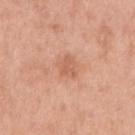Captured during whole-body skin photography for melanoma surveillance; the lesion was not biopsied.
From the right upper arm.
Automated tile analysis of the lesion measured an outline eccentricity of about 0.4 (0 = round, 1 = elongated) and a shape-asymmetry score of about 0.25 (0 = symmetric). The analysis additionally found an average lesion color of about L≈61 a*≈25 b*≈33 (CIELAB), a lesion–skin lightness drop of about 8, and a lesion-to-skin contrast of about 5 (normalized; higher = more distinct). The software also gave an automated nevus-likeness rating near 0 out of 100 and lesion-presence confidence of about 100/100.
Approximately 2.5 mm at its widest.
The tile uses white-light illumination.
A male patient in their 60s.
A 15 mm crop from a total-body photograph taken for skin-cancer surveillance.